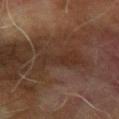  biopsy_status: not biopsied; imaged during a skin examination
  lighting: cross-polarized
  lesion_size:
    long_diameter_mm_approx: 5.0
  site: arm
  patient:
    sex: male
    age_approx: 70
  image:
    source: total-body photography crop
    field_of_view_mm: 15